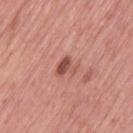A female subject, roughly 55 years of age.
A region of skin cropped from a whole-body photographic capture, roughly 15 mm wide.
About 2.5 mm across.
The lesion is on the leg.
Automated image analysis of the tile measured a lesion area of about 4.5 mm², an eccentricity of roughly 0.6, and two-axis asymmetry of about 0.5. The analysis additionally found a nevus-likeness score of about 10/100 and a lesion-detection confidence of about 100/100.
Captured under white-light illumination.